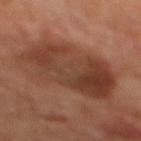Q: Was this lesion biopsied?
A: total-body-photography surveillance lesion; no biopsy